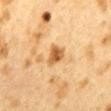Assessment: The lesion was photographed on a routine skin check and not biopsied; there is no pathology result. Image and clinical context: This is a cross-polarized tile. A female subject, aged 38–42. The recorded lesion diameter is about 3 mm. The total-body-photography lesion software estimated a mean CIELAB color near L≈50 a*≈19 b*≈38. Located on the mid back. A 15 mm crop from a total-body photograph taken for skin-cancer surveillance.